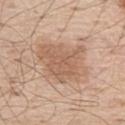The lesion was photographed on a routine skin check and not biopsied; there is no pathology result. The recorded lesion diameter is about 6 mm. A male patient, approximately 70 years of age. On the left thigh. A region of skin cropped from a whole-body photographic capture, roughly 15 mm wide. The tile uses white-light illumination.